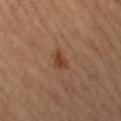{"biopsy_status": "not biopsied; imaged during a skin examination", "patient": {"sex": "female", "age_approx": 35}, "lesion_size": {"long_diameter_mm_approx": 3.0}, "image": {"source": "total-body photography crop", "field_of_view_mm": 15}, "site": "left thigh", "lighting": "cross-polarized"}